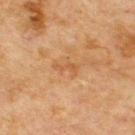Q: Was a biopsy performed?
A: total-body-photography surveillance lesion; no biopsy
Q: What kind of image is this?
A: 15 mm crop, total-body photography
Q: What are the patient's age and sex?
A: male, in their 70s
Q: What is the anatomic site?
A: the back